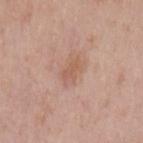Q: Was a biopsy performed?
A: imaged on a skin check; not biopsied
Q: What did automated image analysis measure?
A: a mean CIELAB color near L≈58 a*≈21 b*≈29, roughly 7 lightness units darker than nearby skin, and a lesion-to-skin contrast of about 5.5 (normalized; higher = more distinct); a border-irregularity rating of about 2.5/10, a color-variation rating of about 2.5/10, and a peripheral color-asymmetry measure near 1
Q: How was the tile lit?
A: white-light
Q: How large is the lesion?
A: about 3 mm
Q: What is the anatomic site?
A: the left upper arm
Q: Who is the patient?
A: female, approximately 50 years of age
Q: What kind of image is this?
A: total-body-photography crop, ~15 mm field of view